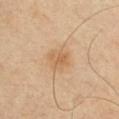| key | value |
|---|---|
| follow-up | total-body-photography surveillance lesion; no biopsy |
| lighting | cross-polarized illumination |
| patient | male, approximately 40 years of age |
| location | the chest |
| imaging modality | ~15 mm tile from a whole-body skin photo |
| lesion size | ≈2.5 mm |
| TBP lesion metrics | a shape eccentricity near 0.6 and a symmetry-axis asymmetry near 0.25; an average lesion color of about L≈61 a*≈19 b*≈37 (CIELAB), a lesion–skin lightness drop of about 8, and a normalized lesion–skin contrast near 5.5; a border-irregularity rating of about 2.5/10 and a peripheral color-asymmetry measure near 1; a lesion-detection confidence of about 100/100 |